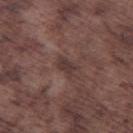  biopsy_status: not biopsied; imaged during a skin examination
  image:
    source: total-body photography crop
    field_of_view_mm: 15
  site: left thigh
  patient:
    sex: male
    age_approx: 75